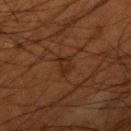Clinical impression: The lesion was tiled from a total-body skin photograph and was not biopsied. Clinical summary: A male subject, aged around 50. Automated image analysis of the tile measured an area of roughly 4 mm², an outline eccentricity of about 0.85 (0 = round, 1 = elongated), and two-axis asymmetry of about 0.3. The analysis additionally found a border-irregularity rating of about 3.5/10, a within-lesion color-variation index near 2.5/10, and a peripheral color-asymmetry measure near 1. It also reported lesion-presence confidence of about 95/100. The lesion is on the right upper arm. Cropped from a total-body skin-imaging series; the visible field is about 15 mm. Measured at roughly 3 mm in maximum diameter.